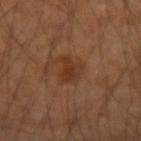Recorded during total-body skin imaging; not selected for excision or biopsy. A male subject, aged 48–52. This is a cross-polarized tile. The lesion is located on the left arm. Cropped from a whole-body photographic skin survey; the tile spans about 15 mm.